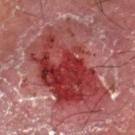{"biopsy_status": "not biopsied; imaged during a skin examination", "automated_metrics": {"area_mm2_approx": 55.0, "eccentricity": 0.7, "shape_asymmetry": 0.35, "vs_skin_darker_L": 11.0, "vs_skin_contrast_norm": 9.5, "border_irregularity_0_10": 5.5, "color_variation_0_10": 9.0, "peripheral_color_asymmetry": 2.5}, "image": {"source": "total-body photography crop", "field_of_view_mm": 15}, "lighting": "cross-polarized", "site": "left thigh", "lesion_size": {"long_diameter_mm_approx": 10.5}, "patient": {"sex": "male", "age_approx": 55}}